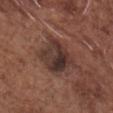Findings:
* biopsy status: catalogued during a skin exam; not biopsied
* site: the chest
* image source: 15 mm crop, total-body photography
* tile lighting: white-light
* patient: male, roughly 75 years of age
* TBP lesion metrics: an average lesion color of about L≈34 a*≈17 b*≈20 (CIELAB), a lesion–skin lightness drop of about 10, and a normalized lesion–skin contrast near 10; a classifier nevus-likeness of about 15/100 and a lesion-detection confidence of about 95/100